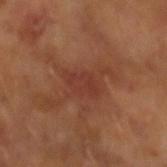Q: Is there a histopathology result?
A: no biopsy performed (imaged during a skin exam)
Q: What is the imaging modality?
A: ~15 mm crop, total-body skin-cancer survey
Q: Illumination type?
A: cross-polarized
Q: Automated lesion metrics?
A: a lesion area of about 6 mm², an outline eccentricity of about 0.8 (0 = round, 1 = elongated), and a symmetry-axis asymmetry near 0.25; a mean CIELAB color near L≈36 a*≈25 b*≈27, a lesion–skin lightness drop of about 5, and a normalized border contrast of about 5.5
Q: Who is the patient?
A: male, aged 63–67